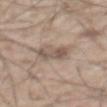Q: Was this lesion biopsied?
A: total-body-photography surveillance lesion; no biopsy
Q: How was this image acquired?
A: ~15 mm crop, total-body skin-cancer survey
Q: What are the patient's age and sex?
A: male, aged approximately 55
Q: Illumination type?
A: white-light illumination
Q: Where on the body is the lesion?
A: the abdomen